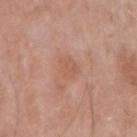Q: Was a biopsy performed?
A: no biopsy performed (imaged during a skin exam)
Q: What are the patient's age and sex?
A: male, aged around 65
Q: What is the imaging modality?
A: ~15 mm crop, total-body skin-cancer survey
Q: Lesion size?
A: about 2.5 mm
Q: What is the anatomic site?
A: the right upper arm
Q: Automated lesion metrics?
A: a border-irregularity rating of about 2.5/10, a color-variation rating of about 2/10, and radial color variation of about 0.5
Q: How was the tile lit?
A: white-light